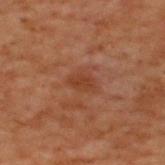{
  "biopsy_status": "not biopsied; imaged during a skin examination",
  "image": {
    "source": "total-body photography crop",
    "field_of_view_mm": 15
  },
  "lesion_size": {
    "long_diameter_mm_approx": 2.5
  },
  "lighting": "cross-polarized",
  "patient": {
    "sex": "male",
    "age_approx": 70
  },
  "site": "upper back",
  "automated_metrics": {
    "eccentricity": 0.8,
    "shape_asymmetry": 0.3,
    "cielab_L": 30,
    "cielab_a": 20,
    "cielab_b": 26,
    "vs_skin_darker_L": 5.0,
    "vs_skin_contrast_norm": 6.0,
    "border_irregularity_0_10": 2.5,
    "color_variation_0_10": 1.0,
    "peripheral_color_asymmetry": 0.5,
    "nevus_likeness_0_100": 5,
    "lesion_detection_confidence_0_100": 100
  }
}